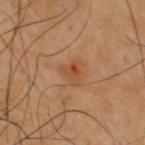Notes:
- workup: total-body-photography surveillance lesion; no biopsy
- site: the upper back
- subject: male, aged 38 to 42
- image source: ~15 mm crop, total-body skin-cancer survey
- automated lesion analysis: an area of roughly 4.5 mm² and an outline eccentricity of about 0.4 (0 = round, 1 = elongated); an average lesion color of about L≈49 a*≈25 b*≈37 (CIELAB), a lesion–skin lightness drop of about 7, and a normalized border contrast of about 6; a classifier nevus-likeness of about 0/100